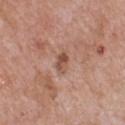The lesion was photographed on a routine skin check and not biopsied; there is no pathology result. Measured at roughly 2.5 mm in maximum diameter. A region of skin cropped from a whole-body photographic capture, roughly 15 mm wide. A male subject aged 58–62. The lesion is on the chest. Captured under white-light illumination.